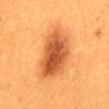The lesion was photographed on a routine skin check and not biopsied; there is no pathology result. The lesion is located on the mid back. The subject is a female aged 28 to 32. Captured under cross-polarized illumination. This image is a 15 mm lesion crop taken from a total-body photograph. The lesion-visualizer software estimated a border-irregularity index near 2.5/10, internal color variation of about 6.5 on a 0–10 scale, and a peripheral color-asymmetry measure near 2. The software also gave a lesion-detection confidence of about 100/100. The recorded lesion diameter is about 7.5 mm.